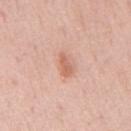Imaged during a routine full-body skin examination; the lesion was not biopsied and no histopathology is available. Automated tile analysis of the lesion measured a footprint of about 3 mm², an eccentricity of roughly 0.9, and a symmetry-axis asymmetry near 0.3. It also reported a lesion-detection confidence of about 100/100. From the mid back. About 2.5 mm across. Captured under white-light illumination. A male subject, aged 58–62. A close-up tile cropped from a whole-body skin photograph, about 15 mm across.